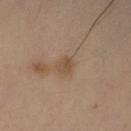Impression: Part of a total-body skin-imaging series; this lesion was reviewed on a skin check and was not flagged for biopsy. Image and clinical context: A 15 mm crop from a total-body photograph taken for skin-cancer surveillance. On the right forearm. About 2.5 mm across. The subject is a female in their mid- to late 40s. Imaged with cross-polarized lighting.